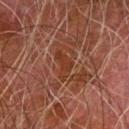Findings:
– biopsy status · no biopsy performed (imaged during a skin exam)
– subject · male, aged 78–82
– image · 15 mm crop, total-body photography
– illumination · cross-polarized illumination
– site · the left forearm
– image-analysis metrics · an average lesion color of about L≈28 a*≈21 b*≈26 (CIELAB) and a normalized lesion–skin contrast near 6.5; a classifier nevus-likeness of about 0/100